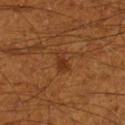Clinical impression: Imaged during a routine full-body skin examination; the lesion was not biopsied and no histopathology is available. Background: On the right lower leg. A male patient aged around 60. The tile uses cross-polarized illumination. An algorithmic analysis of the crop reported a lesion area of about 3.5 mm², an eccentricity of roughly 0.85, and two-axis asymmetry of about 0.35. The software also gave a nevus-likeness score of about 60/100 and a detector confidence of about 100 out of 100 that the crop contains a lesion. A 15 mm close-up tile from a total-body photography series done for melanoma screening.